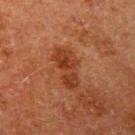Q: Was this lesion biopsied?
A: catalogued during a skin exam; not biopsied
Q: Automated lesion metrics?
A: an area of roughly 9 mm², a shape eccentricity near 0.9, and a symmetry-axis asymmetry near 0.4
Q: How was this image acquired?
A: 15 mm crop, total-body photography
Q: What lighting was used for the tile?
A: cross-polarized
Q: What is the anatomic site?
A: the left upper arm
Q: How large is the lesion?
A: ~5 mm (longest diameter)
Q: Patient demographics?
A: male, approximately 60 years of age